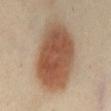Assessment: This lesion was catalogued during total-body skin photography and was not selected for biopsy. Image and clinical context: A female patient, approximately 55 years of age. A 15 mm close-up tile from a total-body photography series done for melanoma screening. From the abdomen. Approximately 10 mm at its widest. The tile uses cross-polarized illumination.